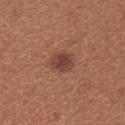This lesion was catalogued during total-body skin photography and was not selected for biopsy.
Located on the left upper arm.
A region of skin cropped from a whole-body photographic capture, roughly 15 mm wide.
Captured under white-light illumination.
A female patient, about 30 years old.